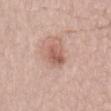Case summary:
– workup: imaged on a skin check; not biopsied
– subject: male, aged around 65
– body site: the lower back
– image: total-body-photography crop, ~15 mm field of view
– automated lesion analysis: a footprint of about 5 mm² and a symmetry-axis asymmetry near 0.3; a border-irregularity index near 3/10, a color-variation rating of about 3/10, and a peripheral color-asymmetry measure near 1.5; an automated nevus-likeness rating near 40 out of 100 and a detector confidence of about 100 out of 100 that the crop contains a lesion
– tile lighting: white-light illumination